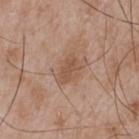biopsy status — imaged on a skin check; not biopsied
imaging modality — 15 mm crop, total-body photography
patient — male, about 65 years old
anatomic site — the chest